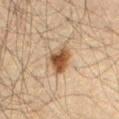Notes:
– anatomic site · the abdomen
– diameter · ~4 mm (longest diameter)
– subject · male, approximately 60 years of age
– imaging modality · ~15 mm tile from a whole-body skin photo